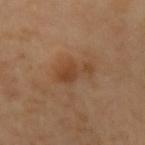Q: Was a biopsy performed?
A: catalogued during a skin exam; not biopsied
Q: What kind of image is this?
A: ~15 mm tile from a whole-body skin photo
Q: Lesion size?
A: about 4 mm
Q: What is the anatomic site?
A: the left upper arm
Q: Automated lesion metrics?
A: a lesion color around L≈44 a*≈21 b*≈34 in CIELAB, about 8 CIELAB-L* units darker than the surrounding skin, and a lesion-to-skin contrast of about 7 (normalized; higher = more distinct); border irregularity of about 5 on a 0–10 scale; a nevus-likeness score of about 70/100 and a lesion-detection confidence of about 100/100
Q: Patient demographics?
A: female, aged 53 to 57
Q: How was the tile lit?
A: cross-polarized illumination